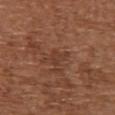Clinical impression: Recorded during total-body skin imaging; not selected for excision or biopsy. Clinical summary: A region of skin cropped from a whole-body photographic capture, roughly 15 mm wide. Captured under white-light illumination. The subject is a female in their mid-70s. The lesion is on the chest. An algorithmic analysis of the crop reported a footprint of about 3.5 mm² and two-axis asymmetry of about 0.45. The lesion's longest dimension is about 3 mm.